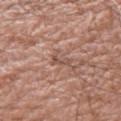The lesion was tiled from a total-body skin photograph and was not biopsied. Located on the leg. A male patient, aged 58–62. Imaged with white-light lighting. Longest diameter approximately 2.5 mm. Cropped from a whole-body photographic skin survey; the tile spans about 15 mm.